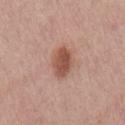Clinical summary:
A male subject aged 73 to 77. The lesion is located on the back. Approximately 4 mm at its widest. A 15 mm close-up extracted from a 3D total-body photography capture.